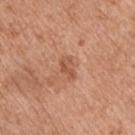body site: the front of the torso
lighting: white-light
size: ≈3 mm
patient: male, about 55 years old
acquisition: ~15 mm tile from a whole-body skin photo